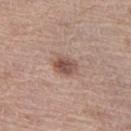Part of a total-body skin-imaging series; this lesion was reviewed on a skin check and was not flagged for biopsy. A female patient about 65 years old. The lesion is on the left thigh. A 15 mm crop from a total-body photograph taken for skin-cancer surveillance.